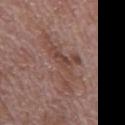This lesion was catalogued during total-body skin photography and was not selected for biopsy.
Automated image analysis of the tile measured a border-irregularity index near 10/10 and a within-lesion color-variation index near 3.5/10.
Captured under white-light illumination.
Approximately 7.5 mm at its widest.
A 15 mm crop from a total-body photograph taken for skin-cancer surveillance.
From the mid back.
A male patient, aged 68 to 72.